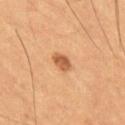notes — catalogued during a skin exam; not biopsied
lighting — cross-polarized illumination
patient — male, aged 58–62
anatomic site — the abdomen
size — ~2.5 mm (longest diameter)
image — 15 mm crop, total-body photography
automated lesion analysis — an average lesion color of about L≈51 a*≈23 b*≈36 (CIELAB); a detector confidence of about 100 out of 100 that the crop contains a lesion